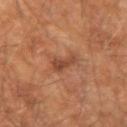Part of a total-body skin-imaging series; this lesion was reviewed on a skin check and was not flagged for biopsy. On the left forearm. The patient is a male roughly 65 years of age. Cropped from a total-body skin-imaging series; the visible field is about 15 mm. Captured under cross-polarized illumination. About 3.5 mm across.